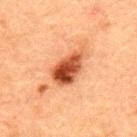{
  "site": "upper back",
  "automated_metrics": {
    "area_mm2_approx": 11.0,
    "shape_asymmetry": 0.3,
    "cielab_L": 42,
    "cielab_a": 26,
    "cielab_b": 33,
    "vs_skin_darker_L": 15.0,
    "vs_skin_contrast_norm": 11.5
  },
  "patient": {
    "sex": "male",
    "age_approx": 50
  },
  "lesion_size": {
    "long_diameter_mm_approx": 5.0
  },
  "image": {
    "source": "total-body photography crop",
    "field_of_view_mm": 15
  },
  "lighting": "cross-polarized"
}